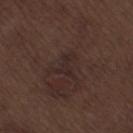Captured during whole-body skin photography for melanoma surveillance; the lesion was not biopsied.
A male patient in their 70s.
A 15 mm close-up extracted from a 3D total-body photography capture.
Located on the right thigh.
The tile uses white-light illumination.